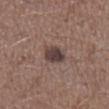notes — catalogued during a skin exam; not biopsied
imaging modality — ~15 mm crop, total-body skin-cancer survey
anatomic site — the leg
subject — male, aged approximately 45